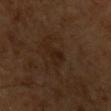Recorded during total-body skin imaging; not selected for excision or biopsy.
On the left upper arm.
Cropped from a whole-body photographic skin survey; the tile spans about 15 mm.
The patient is a male about 60 years old.
Captured under cross-polarized illumination.